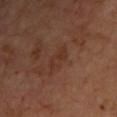Q: Was a biopsy performed?
A: no biopsy performed (imaged during a skin exam)
Q: What are the patient's age and sex?
A: about 65 years old
Q: What is the imaging modality?
A: total-body-photography crop, ~15 mm field of view
Q: What is the anatomic site?
A: the chest